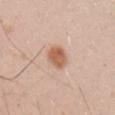The lesion was tiled from a total-body skin photograph and was not biopsied.
A 15 mm close-up tile from a total-body photography series done for melanoma screening.
A male subject aged approximately 30.
The total-body-photography lesion software estimated a lesion area of about 6.5 mm², an eccentricity of roughly 0.7, and a symmetry-axis asymmetry near 0.2. The software also gave a lesion color around L≈60 a*≈22 b*≈33 in CIELAB, roughly 12 lightness units darker than nearby skin, and a lesion-to-skin contrast of about 9 (normalized; higher = more distinct). It also reported a border-irregularity index near 2/10 and a peripheral color-asymmetry measure near 1. The software also gave a lesion-detection confidence of about 100/100.
Longest diameter approximately 3 mm.
The tile uses white-light illumination.
Located on the right upper arm.